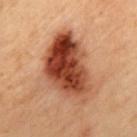{
  "patient": {
    "sex": "male",
    "age_approx": 55
  },
  "site": "mid back",
  "lighting": "cross-polarized",
  "image": {
    "source": "total-body photography crop",
    "field_of_view_mm": 15
  },
  "automated_metrics": {
    "area_mm2_approx": 31.0,
    "eccentricity": 0.8,
    "shape_asymmetry": 0.25,
    "vs_skin_darker_L": 16.0,
    "vs_skin_contrast_norm": 13.0
  }
}